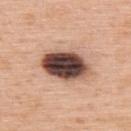{
  "biopsy_status": "not biopsied; imaged during a skin examination",
  "site": "back",
  "image": {
    "source": "total-body photography crop",
    "field_of_view_mm": 15
  },
  "patient": {
    "sex": "male",
    "age_approx": 60
  }
}